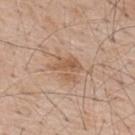| field | value |
|---|---|
| workup | no biopsy performed (imaged during a skin exam) |
| lighting | white-light illumination |
| imaging modality | 15 mm crop, total-body photography |
| subject | male, about 60 years old |
| lesion size | ≈3.5 mm |
| site | the upper back |